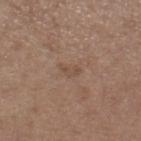notes = total-body-photography surveillance lesion; no biopsy
size = ~2.5 mm (longest diameter)
site = the right lower leg
patient = female, roughly 65 years of age
image source = ~15 mm crop, total-body skin-cancer survey